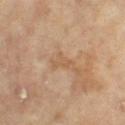{"biopsy_status": "not biopsied; imaged during a skin examination", "image": {"source": "total-body photography crop", "field_of_view_mm": 15}, "lesion_size": {"long_diameter_mm_approx": 3.0}, "patient": {"sex": "female", "age_approx": 75}, "automated_metrics": {"cielab_L": 57, "cielab_a": 18, "cielab_b": 35, "vs_skin_darker_L": 6.0, "vs_skin_contrast_norm": 5.0, "lesion_detection_confidence_0_100": 100}, "lighting": "cross-polarized", "site": "right thigh"}